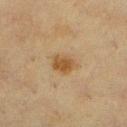No biopsy was performed on this lesion — it was imaged during a full skin examination and was not determined to be concerning. Approximately 3 mm at its widest. A female subject, aged 53 to 57. Automated tile analysis of the lesion measured an area of roughly 6 mm², a shape eccentricity near 0.65, and a shape-asymmetry score of about 0.2 (0 = symmetric). The analysis additionally found about 8 CIELAB-L* units darker than the surrounding skin and a normalized lesion–skin contrast near 8. The analysis additionally found border irregularity of about 2 on a 0–10 scale. The software also gave a detector confidence of about 100 out of 100 that the crop contains a lesion. Captured under cross-polarized illumination. The lesion is located on the left lower leg. Cropped from a whole-body photographic skin survey; the tile spans about 15 mm.